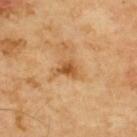Assessment:
Part of a total-body skin-imaging series; this lesion was reviewed on a skin check and was not flagged for biopsy.
Context:
This image is a 15 mm lesion crop taken from a total-body photograph. The tile uses cross-polarized illumination. The recorded lesion diameter is about 2.5 mm. The subject is a male aged 68 to 72. On the upper back.